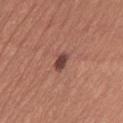workup: catalogued during a skin exam; not biopsied | lighting: white-light illumination | patient: female, aged approximately 55 | location: the left thigh | automated metrics: an average lesion color of about L≈44 a*≈23 b*≈24 (CIELAB), about 13 CIELAB-L* units darker than the surrounding skin, and a lesion-to-skin contrast of about 10 (normalized; higher = more distinct); a border-irregularity index near 3/10, a color-variation rating of about 1.5/10, and radial color variation of about 0.5 | imaging modality: ~15 mm crop, total-body skin-cancer survey | diameter: ~3 mm (longest diameter).